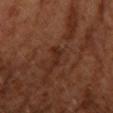<tbp_lesion>
<biopsy_status>not biopsied; imaged during a skin examination</biopsy_status>
</tbp_lesion>